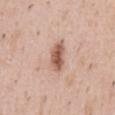Recorded during total-body skin imaging; not selected for excision or biopsy.
Automated image analysis of the tile measured a lesion-to-skin contrast of about 9 (normalized; higher = more distinct). The software also gave a border-irregularity index near 2/10, a within-lesion color-variation index near 4.5/10, and a peripheral color-asymmetry measure near 1.5.
A male subject, aged 38–42.
The lesion is on the abdomen.
Cropped from a total-body skin-imaging series; the visible field is about 15 mm.
The lesion's longest dimension is about 4 mm.